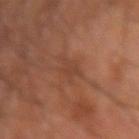Captured during whole-body skin photography for melanoma surveillance; the lesion was not biopsied.
From the arm.
A close-up tile cropped from a whole-body skin photograph, about 15 mm across.
The total-body-photography lesion software estimated an area of roughly 4.5 mm², an eccentricity of roughly 0.85, and a symmetry-axis asymmetry near 0.4. The software also gave an average lesion color of about L≈40 a*≈22 b*≈29 (CIELAB), a lesion–skin lightness drop of about 6, and a normalized lesion–skin contrast near 5. And it measured a nevus-likeness score of about 0/100 and lesion-presence confidence of about 90/100.
The patient is a male roughly 70 years of age.
Captured under cross-polarized illumination.